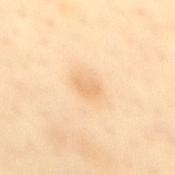Acquisition and patient details:
The subject is a female aged around 45. Longest diameter approximately 2.5 mm. An algorithmic analysis of the crop reported a symmetry-axis asymmetry near 0.2. The analysis additionally found a within-lesion color-variation index near 1.5/10 and radial color variation of about 0.5. It also reported a classifier nevus-likeness of about 40/100. Captured under cross-polarized illumination. A region of skin cropped from a whole-body photographic capture, roughly 15 mm wide. On the mid back.